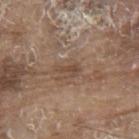workup=no biopsy performed (imaged during a skin exam) | location=the mid back | patient=male, aged 78–82 | imaging modality=~15 mm tile from a whole-body skin photo.